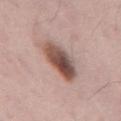Q: Was this lesion biopsied?
A: imaged on a skin check; not biopsied
Q: How was this image acquired?
A: total-body-photography crop, ~15 mm field of view
Q: What are the patient's age and sex?
A: male, about 70 years old
Q: Where on the body is the lesion?
A: the mid back